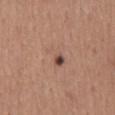Q: How was this image acquired?
A: 15 mm crop, total-body photography
Q: Lesion location?
A: the mid back
Q: Illumination type?
A: white-light
Q: Patient demographics?
A: male, roughly 55 years of age
Q: Lesion size?
A: about 3.5 mm